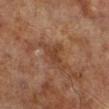The lesion was tiled from a total-body skin photograph and was not biopsied. Approximately 5 mm at its widest. The subject is a male approximately 70 years of age. This is a cross-polarized tile. A 15 mm close-up extracted from a 3D total-body photography capture. The lesion is on the leg.